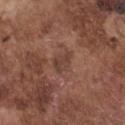Assessment:
Captured during whole-body skin photography for melanoma surveillance; the lesion was not biopsied.
Background:
A lesion tile, about 15 mm wide, cut from a 3D total-body photograph. The lesion is located on the chest. A male patient, aged around 75.